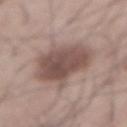This lesion was catalogued during total-body skin photography and was not selected for biopsy.
On the abdomen.
About 6.5 mm across.
A 15 mm close-up extracted from a 3D total-body photography capture.
Imaged with white-light lighting.
A male subject, approximately 55 years of age.